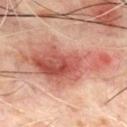Q: Was a biopsy performed?
A: catalogued during a skin exam; not biopsied
Q: What is the imaging modality?
A: total-body-photography crop, ~15 mm field of view
Q: What lighting was used for the tile?
A: cross-polarized illumination
Q: Lesion location?
A: the chest
Q: How large is the lesion?
A: about 11 mm
Q: Patient demographics?
A: male, in their 70s